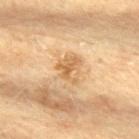Notes:
* biopsy status · total-body-photography surveillance lesion; no biopsy
* patient · female, approximately 80 years of age
* image · ~15 mm crop, total-body skin-cancer survey
* size · ~3 mm (longest diameter)
* location · the upper back
* lighting · cross-polarized illumination
* image-analysis metrics · an automated nevus-likeness rating near 5 out of 100 and a detector confidence of about 100 out of 100 that the crop contains a lesion